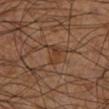Clinical impression: This lesion was catalogued during total-body skin photography and was not selected for biopsy. Clinical summary: From the left lower leg. This image is a 15 mm lesion crop taken from a total-body photograph. A male subject, roughly 60 years of age. The recorded lesion diameter is about 3 mm. Imaged with cross-polarized lighting.